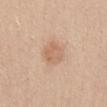Impression:
The lesion was photographed on a routine skin check and not biopsied; there is no pathology result.
Acquisition and patient details:
Captured under white-light illumination. The recorded lesion diameter is about 3 mm. A 15 mm crop from a total-body photograph taken for skin-cancer surveillance. The patient is a female aged 23 to 27. Located on the chest.